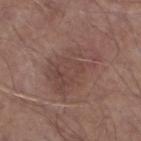This lesion was catalogued during total-body skin photography and was not selected for biopsy. Located on the left lower leg. The subject is a male approximately 65 years of age. About 6.5 mm across. A close-up tile cropped from a whole-body skin photograph, about 15 mm across. The tile uses white-light illumination.